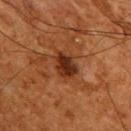{"biopsy_status": "not biopsied; imaged during a skin examination", "patient": {"sex": "male", "age_approx": 65}, "automated_metrics": {"eccentricity": 0.7, "cielab_L": 26, "cielab_a": 21, "cielab_b": 28, "vs_skin_darker_L": 10.0, "vs_skin_contrast_norm": 10.0, "nevus_likeness_0_100": 60, "lesion_detection_confidence_0_100": 100}, "image": {"source": "total-body photography crop", "field_of_view_mm": 15}, "site": "upper back"}